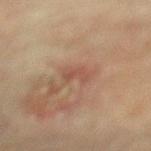Q: Is there a histopathology result?
A: catalogued during a skin exam; not biopsied
Q: Lesion size?
A: ~3 mm (longest diameter)
Q: What kind of image is this?
A: ~15 mm crop, total-body skin-cancer survey
Q: What is the anatomic site?
A: the back
Q: How was the tile lit?
A: cross-polarized illumination
Q: What did automated image analysis measure?
A: roughly 6 lightness units darker than nearby skin and a normalized lesion–skin contrast near 5.5; a border-irregularity rating of about 5.5/10 and radial color variation of about 0; a classifier nevus-likeness of about 0/100
Q: Patient demographics?
A: male, aged 73–77